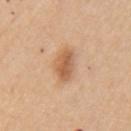<case>
  <biopsy_status>not biopsied; imaged during a skin examination</biopsy_status>
  <image>
    <source>total-body photography crop</source>
    <field_of_view_mm>15</field_of_view_mm>
  </image>
  <patient>
    <sex>female</sex>
    <age_approx>65</age_approx>
  </patient>
  <lighting>white-light</lighting>
  <lesion_size>
    <long_diameter_mm_approx>4.0</long_diameter_mm_approx>
  </lesion_size>
  <site>arm</site>
</case>